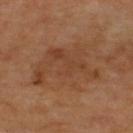Findings:
• notes: total-body-photography surveillance lesion; no biopsy
• subject: aged approximately 65
• location: the back
• acquisition: 15 mm crop, total-body photography
• lesion size: ≈7.5 mm
• lighting: cross-polarized illumination